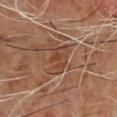Q: Was this lesion biopsied?
A: imaged on a skin check; not biopsied
Q: How large is the lesion?
A: ~4.5 mm (longest diameter)
Q: Lesion location?
A: the chest
Q: What did automated image analysis measure?
A: an area of roughly 8.5 mm² and a symmetry-axis asymmetry near 0.35; a within-lesion color-variation index near 3.5/10 and a peripheral color-asymmetry measure near 1; an automated nevus-likeness rating near 0 out of 100 and lesion-presence confidence of about 65/100
Q: What kind of image is this?
A: ~15 mm crop, total-body skin-cancer survey
Q: Patient demographics?
A: male, aged around 65
Q: Illumination type?
A: cross-polarized illumination